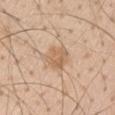workup — imaged on a skin check; not biopsied | site — the right upper arm | tile lighting — white-light | lesion size — ~3 mm (longest diameter) | subject — male, approximately 55 years of age | imaging modality — 15 mm crop, total-body photography.